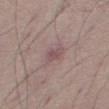This lesion was catalogued during total-body skin photography and was not selected for biopsy. A roughly 15 mm field-of-view crop from a total-body skin photograph. An algorithmic analysis of the crop reported a lesion area of about 4 mm², an eccentricity of roughly 0.8, and a shape-asymmetry score of about 0.2 (0 = symmetric). It also reported an average lesion color of about L≈51 a*≈19 b*≈17 (CIELAB), a lesion–skin lightness drop of about 8, and a normalized border contrast of about 5.5. Imaged with white-light lighting. The lesion is located on the right thigh. Approximately 3 mm at its widest. A male patient, aged approximately 50.